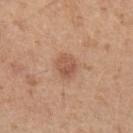Clinical summary:
The recorded lesion diameter is about 3 mm. A roughly 15 mm field-of-view crop from a total-body skin photograph. A female subject aged 38–42. On the left upper arm. The lesion-visualizer software estimated an area of roughly 5.5 mm², a shape eccentricity near 0.6, and a shape-asymmetry score of about 0.2 (0 = symmetric). And it measured a classifier nevus-likeness of about 50/100 and lesion-presence confidence of about 100/100.